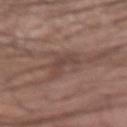Findings:
– subject — male, aged 28 to 32
– site — the right forearm
– acquisition — ~15 mm crop, total-body skin-cancer survey